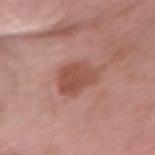{
  "biopsy_status": "not biopsied; imaged during a skin examination",
  "image": {
    "source": "total-body photography crop",
    "field_of_view_mm": 15
  },
  "lesion_size": {
    "long_diameter_mm_approx": 4.0
  },
  "site": "right forearm",
  "patient": {
    "sex": "female",
    "age_approx": 60
  },
  "automated_metrics": {
    "cielab_L": 51,
    "cielab_a": 24,
    "cielab_b": 27,
    "vs_skin_darker_L": 10.0,
    "peripheral_color_asymmetry": 1.0
  },
  "lighting": "white-light"
}